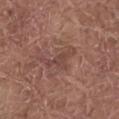Findings:
– notes: catalogued during a skin exam; not biopsied
– subject: male, aged approximately 80
– anatomic site: the abdomen
– imaging modality: ~15 mm crop, total-body skin-cancer survey